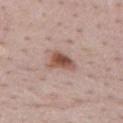Findings:
* diameter — ≈4 mm
* imaging modality — total-body-photography crop, ~15 mm field of view
* patient — male, in their 50s
* anatomic site — the mid back